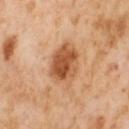Background:
This image is a 15 mm lesion crop taken from a total-body photograph. Imaged with cross-polarized lighting. On the leg. A female subject, aged 53–57. The recorded lesion diameter is about 5 mm.